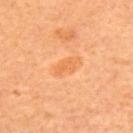workup=imaged on a skin check; not biopsied | location=the back | lighting=cross-polarized | diameter=~3.5 mm (longest diameter) | acquisition=~15 mm crop, total-body skin-cancer survey | patient=female, aged 63 to 67 | TBP lesion metrics=a footprint of about 4.5 mm², a shape eccentricity near 0.85, and two-axis asymmetry of about 0.3; a nevus-likeness score of about 10/100 and a lesion-detection confidence of about 100/100.